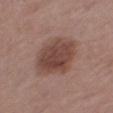The subject is a male about 75 years old.
Captured under white-light illumination.
From the right thigh.
A roughly 15 mm field-of-view crop from a total-body skin photograph.
The lesion-visualizer software estimated a lesion area of about 22 mm², an eccentricity of roughly 0.7, and two-axis asymmetry of about 0.15. The analysis additionally found a lesion–skin lightness drop of about 11 and a normalized border contrast of about 8.